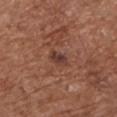Part of a total-body skin-imaging series; this lesion was reviewed on a skin check and was not flagged for biopsy.
A lesion tile, about 15 mm wide, cut from a 3D total-body photograph.
The tile uses white-light illumination.
A female subject aged around 75.
The lesion is located on the chest.
The lesion's longest dimension is about 3 mm.